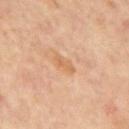{"biopsy_status": "not biopsied; imaged during a skin examination", "site": "mid back", "patient": {"sex": "male", "age_approx": 60}, "image": {"source": "total-body photography crop", "field_of_view_mm": 15}, "automated_metrics": {"area_mm2_approx": 3.5, "eccentricity": 0.9, "border_irregularity_0_10": 3.0, "color_variation_0_10": 2.5, "peripheral_color_asymmetry": 0.5}}